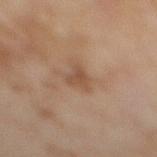follow-up — imaged on a skin check; not biopsied | diameter — about 2.5 mm | imaging modality — total-body-photography crop, ~15 mm field of view | location — the right lower leg | illumination — cross-polarized | patient — female, aged 58 to 62.